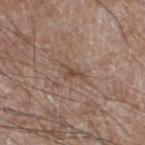workup: no biopsy performed (imaged during a skin exam)
patient: male, in their 60s
diameter: about 3 mm
site: the leg
image source: ~15 mm tile from a whole-body skin photo
illumination: white-light illumination
TBP lesion metrics: a lesion color around L≈48 a*≈16 b*≈26 in CIELAB and a lesion-to-skin contrast of about 6 (normalized; higher = more distinct); an automated nevus-likeness rating near 0 out of 100